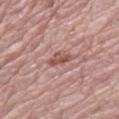Findings:
* biopsy status: imaged on a skin check; not biopsied
* image: 15 mm crop, total-body photography
* illumination: white-light illumination
* subject: female, in their mid- to late 60s
* body site: the right thigh
* TBP lesion metrics: a lesion area of about 4 mm², an eccentricity of roughly 0.8, and a shape-asymmetry score of about 0.3 (0 = symmetric); a lesion color around L≈53 a*≈22 b*≈24 in CIELAB, about 10 CIELAB-L* units darker than the surrounding skin, and a normalized lesion–skin contrast near 7.5; a border-irregularity rating of about 3/10 and a color-variation rating of about 3.5/10; an automated nevus-likeness rating near 0 out of 100 and a lesion-detection confidence of about 100/100
* size: about 3 mm